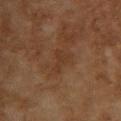follow-up: total-body-photography surveillance lesion; no biopsy.